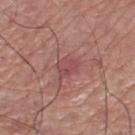{"biopsy_status": "not biopsied; imaged during a skin examination", "patient": {"sex": "male", "age_approx": 70}, "image": {"source": "total-body photography crop", "field_of_view_mm": 15}, "site": "right lower leg"}